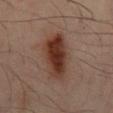The lesion was tiled from a total-body skin photograph and was not biopsied.
A close-up tile cropped from a whole-body skin photograph, about 15 mm across.
Located on the back.
A male patient, aged approximately 50.